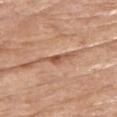Clinical summary: This is a white-light tile. The lesion's longest dimension is about 2.5 mm. A female subject, roughly 75 years of age. This image is a 15 mm lesion crop taken from a total-body photograph. The lesion is on the left upper arm.